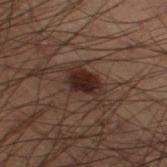Q: Was a biopsy performed?
A: no biopsy performed (imaged during a skin exam)
Q: What did automated image analysis measure?
A: a mean CIELAB color near L≈21 a*≈13 b*≈17, a lesion–skin lightness drop of about 8, and a normalized lesion–skin contrast near 10; a border-irregularity index near 4.5/10, a within-lesion color-variation index near 3/10, and peripheral color asymmetry of about 0.5; an automated nevus-likeness rating near 90 out of 100
Q: Where on the body is the lesion?
A: the right thigh
Q: What kind of image is this?
A: total-body-photography crop, ~15 mm field of view
Q: What are the patient's age and sex?
A: male, about 55 years old
Q: Illumination type?
A: cross-polarized